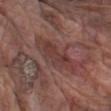Q: Is there a histopathology result?
A: imaged on a skin check; not biopsied
Q: Automated lesion metrics?
A: roughly 7 lightness units darker than nearby skin and a lesion-to-skin contrast of about 6 (normalized; higher = more distinct); a within-lesion color-variation index near 3.5/10 and peripheral color asymmetry of about 1
Q: Where on the body is the lesion?
A: the left upper arm
Q: What are the patient's age and sex?
A: male, approximately 75 years of age
Q: Illumination type?
A: white-light illumination
Q: What is the lesion's diameter?
A: ≈6 mm
Q: What kind of image is this?
A: total-body-photography crop, ~15 mm field of view